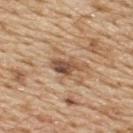Image and clinical context: The lesion is on the upper back. A 15 mm close-up tile from a total-body photography series done for melanoma screening. A male subject aged 68–72.